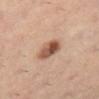| field | value |
|---|---|
| biopsy status | no biopsy performed (imaged during a skin exam) |
| location | the right lower leg |
| image | ~15 mm tile from a whole-body skin photo |
| subject | female, aged around 35 |